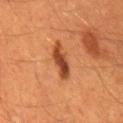  biopsy_status: not biopsied; imaged during a skin examination
  site: back
  lesion_size:
    long_diameter_mm_approx: 5.0
  patient:
    sex: male
    age_approx: 60
  image:
    source: total-body photography crop
    field_of_view_mm: 15
  lighting: cross-polarized